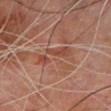Assessment:
No biopsy was performed on this lesion — it was imaged during a full skin examination and was not determined to be concerning.
Background:
A male subject, aged 63–67. Imaged with cross-polarized lighting. This image is a 15 mm lesion crop taken from a total-body photograph. On the chest.